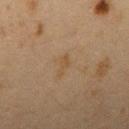Imaged during a routine full-body skin examination; the lesion was not biopsied and no histopathology is available. Imaged with cross-polarized lighting. A 15 mm close-up extracted from a 3D total-body photography capture. The recorded lesion diameter is about 2.5 mm. A female patient aged approximately 40. The lesion is on the right upper arm.